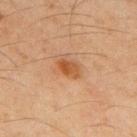{"biopsy_status": "not biopsied; imaged during a skin examination", "lesion_size": {"long_diameter_mm_approx": 3.0}, "image": {"source": "total-body photography crop", "field_of_view_mm": 15}, "site": "upper back", "patient": {"sex": "male", "age_approx": 45}}